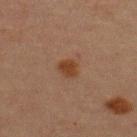Impression:
This lesion was catalogued during total-body skin photography and was not selected for biopsy.
Acquisition and patient details:
The tile uses cross-polarized illumination. An algorithmic analysis of the crop reported a footprint of about 4 mm², a shape eccentricity near 0.5, and a shape-asymmetry score of about 0.25 (0 = symmetric). It also reported a lesion–skin lightness drop of about 7 and a lesion-to-skin contrast of about 8.5 (normalized; higher = more distinct). The analysis additionally found a classifier nevus-likeness of about 95/100 and a detector confidence of about 100 out of 100 that the crop contains a lesion. A lesion tile, about 15 mm wide, cut from a 3D total-body photograph. Located on the upper back. The subject is a male aged 58–62. About 2.5 mm across.